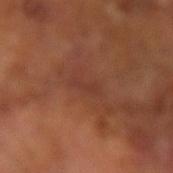The lesion was tiled from a total-body skin photograph and was not biopsied. A male subject, in their mid-60s. Automated image analysis of the tile measured a footprint of about 2.5 mm² and an eccentricity of roughly 0.95. And it measured a lesion color around L≈35 a*≈23 b*≈28 in CIELAB and roughly 5 lightness units darker than nearby skin. The software also gave a border-irregularity rating of about 4.5/10, a color-variation rating of about 0/10, and peripheral color asymmetry of about 0. On the left arm. Cropped from a total-body skin-imaging series; the visible field is about 15 mm.